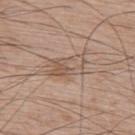Background: Captured under white-light illumination. Located on the upper back. The patient is a male aged 63–67. Measured at roughly 4.5 mm in maximum diameter. The total-body-photography lesion software estimated a lesion area of about 9 mm² and an eccentricity of roughly 0.8. The software also gave an average lesion color of about L≈55 a*≈16 b*≈27 (CIELAB) and a lesion–skin lightness drop of about 8. A lesion tile, about 15 mm wide, cut from a 3D total-body photograph.